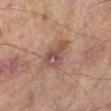Assessment:
The lesion was photographed on a routine skin check and not biopsied; there is no pathology result.
Acquisition and patient details:
A roughly 15 mm field-of-view crop from a total-body skin photograph. The recorded lesion diameter is about 5.5 mm. The lesion is on the left lower leg. Imaged with cross-polarized lighting. The subject is a male roughly 65 years of age.